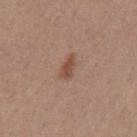{
  "biopsy_status": "not biopsied; imaged during a skin examination",
  "patient": {
    "sex": "female",
    "age_approx": 55
  },
  "lighting": "white-light",
  "image": {
    "source": "total-body photography crop",
    "field_of_view_mm": 15
  },
  "lesion_size": {
    "long_diameter_mm_approx": 3.0
  },
  "site": "mid back"
}